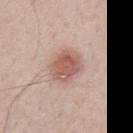Impression:
No biopsy was performed on this lesion — it was imaged during a full skin examination and was not determined to be concerning.
Image and clinical context:
The patient is a male approximately 40 years of age. A lesion tile, about 15 mm wide, cut from a 3D total-body photograph. From the back. About 4.5 mm across. Captured under white-light illumination. Automated tile analysis of the lesion measured a footprint of about 11 mm², an eccentricity of roughly 0.7, and a symmetry-axis asymmetry near 0.15. The software also gave about 12 CIELAB-L* units darker than the surrounding skin and a normalized border contrast of about 7.5. The software also gave a within-lesion color-variation index near 4/10 and peripheral color asymmetry of about 1.5.